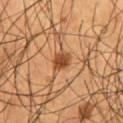{"biopsy_status": "not biopsied; imaged during a skin examination", "patient": {"sex": "male", "age_approx": 55}, "image": {"source": "total-body photography crop", "field_of_view_mm": 15}, "lesion_size": {"long_diameter_mm_approx": 2.5}, "automated_metrics": {"area_mm2_approx": 4.0, "eccentricity": 0.65, "shape_asymmetry": 0.15, "border_irregularity_0_10": 1.5, "color_variation_0_10": 3.5, "peripheral_color_asymmetry": 1.5}, "site": "abdomen"}